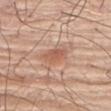Part of a total-body skin-imaging series; this lesion was reviewed on a skin check and was not flagged for biopsy.
The patient is a male approximately 75 years of age.
A 15 mm close-up tile from a total-body photography series done for melanoma screening.
Located on the chest.